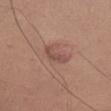The lesion was photographed on a routine skin check and not biopsied; there is no pathology result. On the leg. A male subject, aged approximately 40. This image is a 15 mm lesion crop taken from a total-body photograph. Captured under white-light illumination.